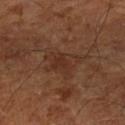The lesion's longest dimension is about 4 mm.
Cropped from a whole-body photographic skin survey; the tile spans about 15 mm.
The patient is approximately 65 years of age.
An algorithmic analysis of the crop reported a nevus-likeness score of about 0/100.
Imaged with cross-polarized lighting.
Located on the left lower leg.